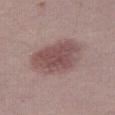The lesion was photographed on a routine skin check and not biopsied; there is no pathology result.
A 15 mm close-up tile from a total-body photography series done for melanoma screening.
From the right thigh.
The patient is a female about 40 years old.